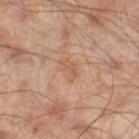{"biopsy_status": "not biopsied; imaged during a skin examination", "image": {"source": "total-body photography crop", "field_of_view_mm": 15}, "lighting": "cross-polarized", "patient": {"sex": "male", "age_approx": 65}, "lesion_size": {"long_diameter_mm_approx": 3.0}, "site": "leg"}